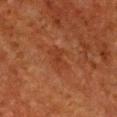Captured during whole-body skin photography for melanoma surveillance; the lesion was not biopsied. This is a cross-polarized tile. A 15 mm close-up tile from a total-body photography series done for melanoma screening. A female subject, aged 48 to 52. The lesion is on the chest. Measured at roughly 3.5 mm in maximum diameter.